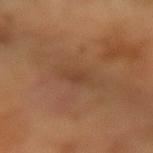Impression:
Captured during whole-body skin photography for melanoma surveillance; the lesion was not biopsied.
Image and clinical context:
A 15 mm close-up tile from a total-body photography series done for melanoma screening. Measured at roughly 3 mm in maximum diameter. From the right forearm. A female subject, aged around 55. The lesion-visualizer software estimated a classifier nevus-likeness of about 0/100 and a lesion-detection confidence of about 100/100.